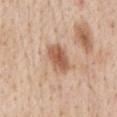The lesion was photographed on a routine skin check and not biopsied; there is no pathology result.
Longest diameter approximately 4 mm.
A male patient, approximately 60 years of age.
This is a white-light tile.
A 15 mm close-up tile from a total-body photography series done for melanoma screening.
The lesion is located on the abdomen.